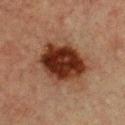<case>
  <biopsy_status>not biopsied; imaged during a skin examination</biopsy_status>
  <site>chest</site>
  <image>
    <source>total-body photography crop</source>
    <field_of_view_mm>15</field_of_view_mm>
  </image>
  <lesion_size>
    <long_diameter_mm_approx>6.0</long_diameter_mm_approx>
  </lesion_size>
  <patient>
    <sex>female</sex>
    <age_approx>55</age_approx>
  </patient>
  <automated_metrics>
    <eccentricity>0.65</eccentricity>
    <shape_asymmetry>0.15</shape_asymmetry>
    <cielab_L>25</cielab_L>
    <cielab_a>21</cielab_a>
    <cielab_b>25</cielab_b>
    <vs_skin_darker_L>16.0</vs_skin_darker_L>
    <border_irregularity_0_10>1.5</border_irregularity_0_10>
    <color_variation_0_10>5.5</color_variation_0_10>
  </automated_metrics>
</case>